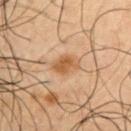A male subject, roughly 50 years of age. Located on the arm. Automated image analysis of the tile measured about 8 CIELAB-L* units darker than the surrounding skin and a lesion-to-skin contrast of about 7.5 (normalized; higher = more distinct). The analysis additionally found lesion-presence confidence of about 100/100. This image is a 15 mm lesion crop taken from a total-body photograph.